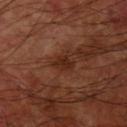Clinical impression:
Recorded during total-body skin imaging; not selected for excision or biopsy.
Background:
A male subject about 60 years old. Located on the right upper arm. Cropped from a total-body skin-imaging series; the visible field is about 15 mm.